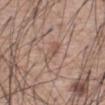Q: Is there a histopathology result?
A: catalogued during a skin exam; not biopsied
Q: What is the anatomic site?
A: the abdomen
Q: What did automated image analysis measure?
A: a border-irregularity index near 2.5/10, a color-variation rating of about 2.5/10, and a peripheral color-asymmetry measure near 1; a classifier nevus-likeness of about 0/100 and lesion-presence confidence of about 90/100
Q: What kind of image is this?
A: ~15 mm tile from a whole-body skin photo
Q: What are the patient's age and sex?
A: male, roughly 60 years of age
Q: Lesion size?
A: about 3.5 mm
Q: How was the tile lit?
A: white-light